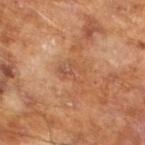Q: What is the imaging modality?
A: ~15 mm tile from a whole-body skin photo
Q: What are the patient's age and sex?
A: male, aged 63 to 67